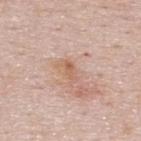Clinical impression:
Captured during whole-body skin photography for melanoma surveillance; the lesion was not biopsied.
Clinical summary:
A female patient aged 48 to 52. From the back. A 15 mm close-up tile from a total-body photography series done for melanoma screening. This is a white-light tile. The recorded lesion diameter is about 2.5 mm.